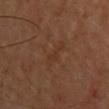notes — imaged on a skin check; not biopsied | illumination — cross-polarized | lesion size — about 4.5 mm | acquisition — ~15 mm tile from a whole-body skin photo | anatomic site — the head or neck | subject — female, approximately 40 years of age | image-analysis metrics — a shape eccentricity near 0.95 and two-axis asymmetry of about 0.4; a border-irregularity index near 6/10, a within-lesion color-variation index near 0/10, and radial color variation of about 0.